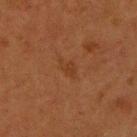biopsy status — no biopsy performed (imaged during a skin exam)
illumination — cross-polarized
automated metrics — a border-irregularity index near 3.5/10, internal color variation of about 1 on a 0–10 scale, and peripheral color asymmetry of about 0.5; an automated nevus-likeness rating near 0 out of 100 and a lesion-detection confidence of about 100/100
acquisition — ~15 mm tile from a whole-body skin photo
subject — female, aged around 40
size — ~2.5 mm (longest diameter)
body site — the upper back